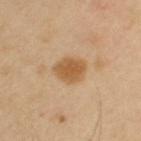Captured during whole-body skin photography for melanoma surveillance; the lesion was not biopsied.
Cropped from a total-body skin-imaging series; the visible field is about 15 mm.
The subject is a female in their 40s.
Imaged with cross-polarized lighting.
The lesion is located on the left upper arm.